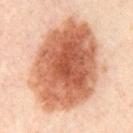follow-up — catalogued during a skin exam; not biopsied | automated metrics — a footprint of about 65 mm², an outline eccentricity of about 0.7 (0 = round, 1 = elongated), and a shape-asymmetry score of about 0.1 (0 = symmetric); a mean CIELAB color near L≈61 a*≈26 b*≈35 and a normalized border contrast of about 11.5; a border-irregularity rating of about 1.5/10, a color-variation rating of about 7.5/10, and a peripheral color-asymmetry measure near 2; an automated nevus-likeness rating near 100 out of 100 and a lesion-detection confidence of about 100/100 | image source — total-body-photography crop, ~15 mm field of view | anatomic site — the front of the torso | lighting — cross-polarized illumination | lesion diameter — ~11 mm (longest diameter) | patient — in their mid-50s.